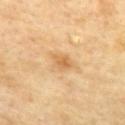Captured during whole-body skin photography for melanoma surveillance; the lesion was not biopsied. A female subject, aged around 75. The tile uses cross-polarized illumination. The lesion is on the front of the torso. A close-up tile cropped from a whole-body skin photograph, about 15 mm across. The lesion's longest dimension is about 2.5 mm.